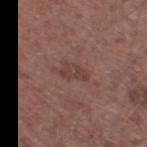<tbp_lesion>
<biopsy_status>not biopsied; imaged during a skin examination</biopsy_status>
<site>leg</site>
<lesion_size>
  <long_diameter_mm_approx>3.0</long_diameter_mm_approx>
</lesion_size>
<image>
  <source>total-body photography crop</source>
  <field_of_view_mm>15</field_of_view_mm>
</image>
<lighting>white-light</lighting>
<patient>
  <sex>male</sex>
  <age_approx>60</age_approx>
</patient>
</tbp_lesion>